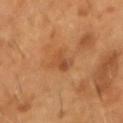This lesion was catalogued during total-body skin photography and was not selected for biopsy.
The subject is a male roughly 55 years of age.
About 3.5 mm across.
This is a cross-polarized tile.
On the head or neck.
Cropped from a whole-body photographic skin survey; the tile spans about 15 mm.
The lesion-visualizer software estimated an outline eccentricity of about 0.85 (0 = round, 1 = elongated) and a shape-asymmetry score of about 0.35 (0 = symmetric). And it measured a lesion color around L≈45 a*≈23 b*≈35 in CIELAB, a lesion–skin lightness drop of about 7, and a normalized border contrast of about 5.5. The software also gave a peripheral color-asymmetry measure near 2.